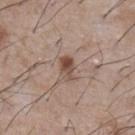Assessment: The lesion was photographed on a routine skin check and not biopsied; there is no pathology result. Clinical summary: The tile uses white-light illumination. A male patient approximately 75 years of age. This image is a 15 mm lesion crop taken from a total-body photograph. On the chest. The lesion-visualizer software estimated a footprint of about 4.5 mm², an outline eccentricity of about 0.8 (0 = round, 1 = elongated), and two-axis asymmetry of about 0.25. And it measured a border-irregularity rating of about 2.5/10, a within-lesion color-variation index near 7/10, and radial color variation of about 3.